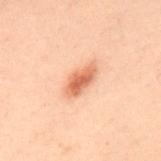* biopsy status · total-body-photography surveillance lesion; no biopsy
* lesion size · about 4 mm
* tile lighting · cross-polarized
* anatomic site · the upper back
* imaging modality · 15 mm crop, total-body photography
* patient · male, about 40 years old